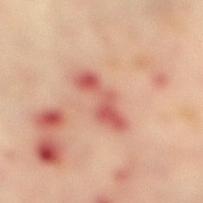Assessment:
The lesion was tiled from a total-body skin photograph and was not biopsied.
Context:
A 15 mm close-up tile from a total-body photography series done for melanoma screening. An algorithmic analysis of the crop reported an area of roughly 8.5 mm². The analysis additionally found a lesion-detection confidence of about 100/100. The recorded lesion diameter is about 5.5 mm. A female patient roughly 65 years of age. This is a cross-polarized tile. From the right lower leg.